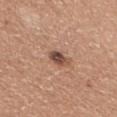Q: Was a biopsy performed?
A: no biopsy performed (imaged during a skin exam)
Q: Lesion location?
A: the right upper arm
Q: What is the lesion's diameter?
A: ≈3 mm
Q: What is the imaging modality?
A: total-body-photography crop, ~15 mm field of view
Q: Who is the patient?
A: female, aged 53 to 57